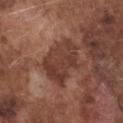Case summary:
* notes: no biopsy performed (imaged during a skin exam)
* body site: the chest
* TBP lesion metrics: internal color variation of about 4 on a 0–10 scale and radial color variation of about 1.5; an automated nevus-likeness rating near 0 out of 100
* image source: ~15 mm tile from a whole-body skin photo
* patient: male, roughly 75 years of age